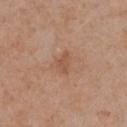Assessment:
Imaged during a routine full-body skin examination; the lesion was not biopsied and no histopathology is available.
Background:
The tile uses white-light illumination. A region of skin cropped from a whole-body photographic capture, roughly 15 mm wide. The lesion-visualizer software estimated an area of roughly 3.5 mm² and an outline eccentricity of about 0.75 (0 = round, 1 = elongated). The analysis additionally found about 7 CIELAB-L* units darker than the surrounding skin and a normalized border contrast of about 5.5. The software also gave a border-irregularity rating of about 4/10, a within-lesion color-variation index near 1/10, and peripheral color asymmetry of about 0.5. The lesion is on the chest. A female patient about 55 years old. The lesion's longest dimension is about 2.5 mm.